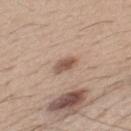No biopsy was performed on this lesion — it was imaged during a full skin examination and was not determined to be concerning.